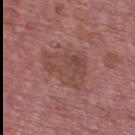Q: Was this lesion biopsied?
A: no biopsy performed (imaged during a skin exam)
Q: What are the patient's age and sex?
A: male, about 75 years old
Q: How was this image acquired?
A: ~15 mm tile from a whole-body skin photo
Q: What is the anatomic site?
A: the upper back
Q: What is the lesion's diameter?
A: ~5.5 mm (longest diameter)